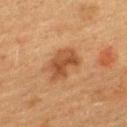Clinical impression:
Captured during whole-body skin photography for melanoma surveillance; the lesion was not biopsied.
Image and clinical context:
On the upper back. The patient is a female aged 53–57. The tile uses cross-polarized illumination. A 15 mm crop from a total-body photograph taken for skin-cancer surveillance. The total-body-photography lesion software estimated a footprint of about 9.5 mm², an outline eccentricity of about 0.75 (0 = round, 1 = elongated), and a shape-asymmetry score of about 0.25 (0 = symmetric). The software also gave a lesion color around L≈40 a*≈20 b*≈32 in CIELAB and a lesion-to-skin contrast of about 8 (normalized; higher = more distinct). It also reported a detector confidence of about 100 out of 100 that the crop contains a lesion. Longest diameter approximately 4.5 mm.